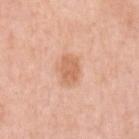Case summary:
- patient · female, about 50 years old
- illumination · white-light illumination
- size · about 3 mm
- imaging modality · 15 mm crop, total-body photography
- site · the left upper arm